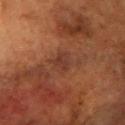<record>
<lesion_size>
  <long_diameter_mm_approx>3.0</long_diameter_mm_approx>
</lesion_size>
<patient>
  <sex>female</sex>
  <age_approx>80</age_approx>
</patient>
<image>
  <source>total-body photography crop</source>
  <field_of_view_mm>15</field_of_view_mm>
</image>
<automated_metrics>
  <eccentricity>0.65</eccentricity>
  <color_variation_0_10>2.0</color_variation_0_10>
  <peripheral_color_asymmetry>1.0</peripheral_color_asymmetry>
  <nevus_likeness_0_100>5</nevus_likeness_0_100>
  <lesion_detection_confidence_0_100>95</lesion_detection_confidence_0_100>
</automated_metrics>
<lighting>cross-polarized</lighting>
<site>head or neck</site>
</record>